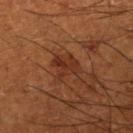Assessment:
Captured during whole-body skin photography for melanoma surveillance; the lesion was not biopsied.
Image and clinical context:
A male subject in their mid- to late 60s. Automated image analysis of the tile measured an area of roughly 7 mm², an outline eccentricity of about 0.6 (0 = round, 1 = elongated), and a symmetry-axis asymmetry near 0.35. The analysis additionally found a mean CIELAB color near L≈33 a*≈25 b*≈32, a lesion–skin lightness drop of about 7, and a lesion-to-skin contrast of about 6.5 (normalized; higher = more distinct). The software also gave a border-irregularity rating of about 3.5/10, a color-variation rating of about 4/10, and radial color variation of about 1.5. And it measured a nevus-likeness score of about 5/100 and a detector confidence of about 100 out of 100 that the crop contains a lesion. Located on the right lower leg. A 15 mm close-up extracted from a 3D total-body photography capture.